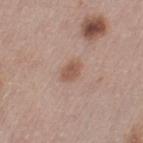Part of a total-body skin-imaging series; this lesion was reviewed on a skin check and was not flagged for biopsy. The patient is a female in their mid- to late 60s. Captured under white-light illumination. Located on the left thigh. Longest diameter approximately 2.5 mm. A region of skin cropped from a whole-body photographic capture, roughly 15 mm wide.